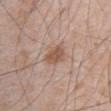biopsy status — imaged on a skin check; not biopsied
patient — male, aged around 65
site — the abdomen
lighting — white-light illumination
image source — ~15 mm tile from a whole-body skin photo
lesion diameter — about 3 mm
TBP lesion metrics — a mean CIELAB color near L≈54 a*≈20 b*≈28, roughly 10 lightness units darker than nearby skin, and a normalized border contrast of about 7.5; border irregularity of about 2 on a 0–10 scale, a within-lesion color-variation index near 1.5/10, and peripheral color asymmetry of about 0.5